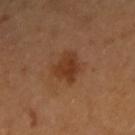Q: Was a biopsy performed?
A: catalogued during a skin exam; not biopsied
Q: What are the patient's age and sex?
A: female, aged approximately 55
Q: What did automated image analysis measure?
A: a color-variation rating of about 2.5/10 and peripheral color asymmetry of about 1; a nevus-likeness score of about 90/100
Q: Where on the body is the lesion?
A: the right forearm
Q: Illumination type?
A: cross-polarized illumination
Q: What is the imaging modality?
A: ~15 mm crop, total-body skin-cancer survey